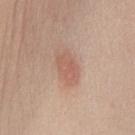Q: Is there a histopathology result?
A: no biopsy performed (imaged during a skin exam)
Q: Where on the body is the lesion?
A: the chest
Q: What is the lesion's diameter?
A: about 4 mm
Q: What kind of image is this?
A: total-body-photography crop, ~15 mm field of view
Q: What are the patient's age and sex?
A: female, aged around 40
Q: Illumination type?
A: white-light